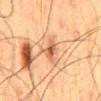Q: Was this lesion biopsied?
A: catalogued during a skin exam; not biopsied
Q: What is the anatomic site?
A: the mid back
Q: How large is the lesion?
A: about 3 mm
Q: What is the imaging modality?
A: ~15 mm tile from a whole-body skin photo
Q: Illumination type?
A: cross-polarized
Q: Who is the patient?
A: male, approximately 60 years of age